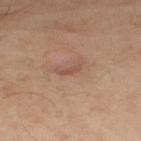{"biopsy_status": "not biopsied; imaged during a skin examination", "site": "upper back", "lesion_size": {"long_diameter_mm_approx": 2.5}, "patient": {"sex": "male", "age_approx": 50}, "image": {"source": "total-body photography crop", "field_of_view_mm": 15}}